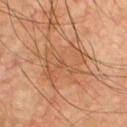Acquisition and patient details:
A 15 mm close-up extracted from a 3D total-body photography capture. Located on the chest. The subject is a male roughly 60 years of age. Measured at roughly 8 mm in maximum diameter. Imaged with cross-polarized lighting. Automated image analysis of the tile measured a lesion color around L≈55 a*≈22 b*≈36 in CIELAB, about 7 CIELAB-L* units darker than the surrounding skin, and a normalized lesion–skin contrast near 5. It also reported an automated nevus-likeness rating near 0 out of 100.